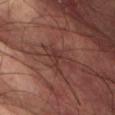On the left thigh.
A 15 mm crop from a total-body photograph taken for skin-cancer surveillance.
Imaged with cross-polarized lighting.
The lesion-visualizer software estimated a footprint of about 5 mm², an eccentricity of roughly 0.8, and two-axis asymmetry of about 0.55. And it measured an average lesion color of about L≈31 a*≈19 b*≈20 (CIELAB) and a lesion–skin lightness drop of about 6.
The patient is a male aged 63–67.
Longest diameter approximately 3.5 mm.